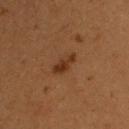Captured during whole-body skin photography for melanoma surveillance; the lesion was not biopsied.
A male subject in their 50s.
Imaged with cross-polarized lighting.
Longest diameter approximately 3.5 mm.
A region of skin cropped from a whole-body photographic capture, roughly 15 mm wide.
On the chest.